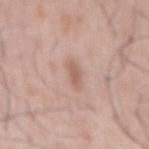  biopsy_status: not biopsied; imaged during a skin examination
  lighting: white-light
  automated_metrics:
    area_mm2_approx: 3.5
    eccentricity: 0.9
    shape_asymmetry: 0.4
    cielab_L: 59
    cielab_a: 19
    cielab_b: 26
    vs_skin_darker_L: 9.0
    vs_skin_contrast_norm: 6.0
    border_irregularity_0_10: 3.5
    color_variation_0_10: 0.5
    peripheral_color_asymmetry: 0.0
  site: mid back
  image:
    source: total-body photography crop
    field_of_view_mm: 15
  patient:
    sex: male
    age_approx: 55
  lesion_size:
    long_diameter_mm_approx: 3.0